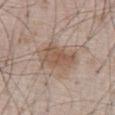biopsy_status: not biopsied; imaged during a skin examination
patient:
  sex: male
  age_approx: 65
image:
  source: total-body photography crop
  field_of_view_mm: 15
lesion_size:
  long_diameter_mm_approx: 5.5
site: abdomen
lighting: white-light